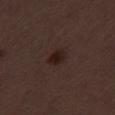Clinical impression:
No biopsy was performed on this lesion — it was imaged during a full skin examination and was not determined to be concerning.
Clinical summary:
The subject is a female aged 48–52. Captured under white-light illumination. The lesion is on the left thigh. An algorithmic analysis of the crop reported an area of roughly 4 mm², an outline eccentricity of about 0.7 (0 = round, 1 = elongated), and two-axis asymmetry of about 0.3. It also reported a classifier nevus-likeness of about 90/100 and lesion-presence confidence of about 100/100. About 2.5 mm across. A region of skin cropped from a whole-body photographic capture, roughly 15 mm wide.